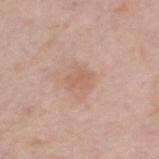<case>
<biopsy_status>not biopsied; imaged during a skin examination</biopsy_status>
<image>
  <source>total-body photography crop</source>
  <field_of_view_mm>15</field_of_view_mm>
</image>
<site>leg</site>
<lesion_size>
  <long_diameter_mm_approx>2.5</long_diameter_mm_approx>
</lesion_size>
<patient>
  <sex>female</sex>
  <age_approx>55</age_approx>
</patient>
</case>